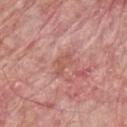Clinical summary:
Captured under white-light illumination. Located on the chest. The lesion's longest dimension is about 3 mm. This image is a 15 mm lesion crop taken from a total-body photograph. The patient is a male about 65 years old.